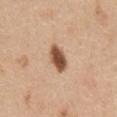Case summary:
– follow-up · no biopsy performed (imaged during a skin exam)
– lesion size · about 4 mm
– lighting · white-light
– image · ~15 mm tile from a whole-body skin photo
– automated lesion analysis · a footprint of about 6.5 mm² and a shape eccentricity near 0.85; a mean CIELAB color near L≈53 a*≈21 b*≈32 and a lesion-to-skin contrast of about 12 (normalized; higher = more distinct)
– patient · female, in their mid-40s
– body site · the chest